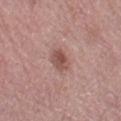No biopsy was performed on this lesion — it was imaged during a full skin examination and was not determined to be concerning. From the leg. Approximately 3 mm at its widest. Automated tile analysis of the lesion measured a footprint of about 4.5 mm², a shape eccentricity near 0.8, and a shape-asymmetry score of about 0.2 (0 = symmetric). The analysis additionally found border irregularity of about 2 on a 0–10 scale, a color-variation rating of about 3.5/10, and a peripheral color-asymmetry measure near 1. The software also gave an automated nevus-likeness rating near 70 out of 100 and a lesion-detection confidence of about 100/100. A female subject, approximately 65 years of age. A 15 mm crop from a total-body photograph taken for skin-cancer surveillance. The tile uses white-light illumination.